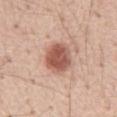Imaged during a routine full-body skin examination; the lesion was not biopsied and no histopathology is available. A close-up tile cropped from a whole-body skin photograph, about 15 mm across. Imaged with white-light lighting. Longest diameter approximately 4 mm. A male subject aged approximately 55. The lesion is on the abdomen.